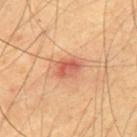The lesion was photographed on a routine skin check and not biopsied; there is no pathology result. A 15 mm crop from a total-body photograph taken for skin-cancer surveillance. Automated tile analysis of the lesion measured a lesion color around L≈60 a*≈29 b*≈35 in CIELAB, roughly 11 lightness units darker than nearby skin, and a lesion-to-skin contrast of about 7 (normalized; higher = more distinct). It also reported border irregularity of about 2 on a 0–10 scale, a within-lesion color-variation index near 6.5/10, and a peripheral color-asymmetry measure near 2.5. Imaged with cross-polarized lighting. The subject is a male approximately 65 years of age. The lesion is on the mid back.